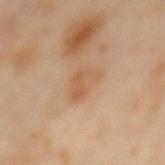{"biopsy_status": "not biopsied; imaged during a skin examination", "lesion_size": {"long_diameter_mm_approx": 4.0}, "site": "back", "patient": {"sex": "male", "age_approx": 65}, "lighting": "cross-polarized", "automated_metrics": {"cielab_L": 58, "cielab_a": 19, "cielab_b": 35, "vs_skin_darker_L": 7.0, "vs_skin_contrast_norm": 5.5, "color_variation_0_10": 3.0}, "image": {"source": "total-body photography crop", "field_of_view_mm": 15}}